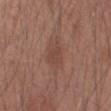Impression: The lesion was photographed on a routine skin check and not biopsied; there is no pathology result. Image and clinical context: Automated image analysis of the tile measured a footprint of about 5.5 mm² and a symmetry-axis asymmetry near 0.25. The analysis additionally found a mean CIELAB color near L≈44 a*≈20 b*≈25, about 6 CIELAB-L* units darker than the surrounding skin, and a lesion-to-skin contrast of about 5 (normalized; higher = more distinct). And it measured a within-lesion color-variation index near 1.5/10. The lesion's longest dimension is about 3 mm. A lesion tile, about 15 mm wide, cut from a 3D total-body photograph. Imaged with white-light lighting. A male subject in their mid- to late 40s. From the left upper arm.